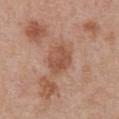The recorded lesion diameter is about 4 mm. The total-body-photography lesion software estimated a classifier nevus-likeness of about 0/100 and a detector confidence of about 100 out of 100 that the crop contains a lesion. The tile uses white-light illumination. The patient is a male aged 68 to 72. The lesion is located on the front of the torso. A 15 mm crop from a total-body photograph taken for skin-cancer surveillance.